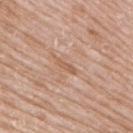The lesion was photographed on a routine skin check and not biopsied; there is no pathology result.
A female subject aged 53 to 57.
A close-up tile cropped from a whole-body skin photograph, about 15 mm across.
On the right upper arm.
The lesion's longest dimension is about 3 mm.
Imaged with white-light lighting.
Automated tile analysis of the lesion measured a lesion color around L≈58 a*≈20 b*≈32 in CIELAB, a lesion–skin lightness drop of about 8, and a lesion-to-skin contrast of about 5.5 (normalized; higher = more distinct). It also reported a border-irregularity rating of about 4.5/10 and peripheral color asymmetry of about 0.